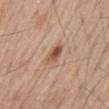Context: The patient is a male aged around 70. Automated image analysis of the tile measured an area of roughly 4 mm², a shape eccentricity near 0.75, and a symmetry-axis asymmetry near 0.2. The analysis additionally found a color-variation rating of about 6/10 and radial color variation of about 2. The lesion is on the mid back. Imaged with white-light lighting. A roughly 15 mm field-of-view crop from a total-body skin photograph. The lesion's longest dimension is about 2.5 mm.